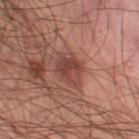notes: no biopsy performed (imaged during a skin exam)
anatomic site: the left lower leg
lighting: cross-polarized
imaging modality: 15 mm crop, total-body photography
subject: male, aged around 40
TBP lesion metrics: a lesion color around L≈42 a*≈24 b*≈23 in CIELAB, roughly 9 lightness units darker than nearby skin, and a lesion-to-skin contrast of about 7.5 (normalized; higher = more distinct); a border-irregularity rating of about 2.5/10 and radial color variation of about 1.5
lesion diameter: about 4 mm